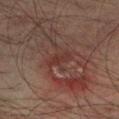Clinical impression: The lesion was photographed on a routine skin check and not biopsied; there is no pathology result. Acquisition and patient details: The subject is a male approximately 75 years of age. A 15 mm close-up extracted from a 3D total-body photography capture. Located on the left lower leg.